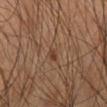Imaged during a routine full-body skin examination; the lesion was not biopsied and no histopathology is available.
A 15 mm close-up tile from a total-body photography series done for melanoma screening.
On the right lower leg.
Imaged with cross-polarized lighting.
The subject is a male about 50 years old.